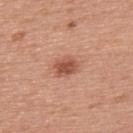follow-up: imaged on a skin check; not biopsied | site: the back | subject: male, about 50 years old | image: ~15 mm crop, total-body skin-cancer survey.